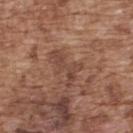follow-up: no biopsy performed (imaged during a skin exam); subject: male, in their mid- to late 70s; diameter: ≈4.5 mm; acquisition: ~15 mm crop, total-body skin-cancer survey; anatomic site: the upper back.